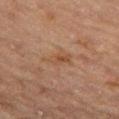This lesion was catalogued during total-body skin photography and was not selected for biopsy.
On the left thigh.
A female subject aged 53–57.
The lesion-visualizer software estimated an area of roughly 5.5 mm² and a shape eccentricity near 0.8. The analysis additionally found a lesion color around L≈43 a*≈17 b*≈29 in CIELAB, a lesion–skin lightness drop of about 5, and a normalized border contrast of about 5.5. It also reported a nevus-likeness score of about 0/100.
A region of skin cropped from a whole-body photographic capture, roughly 15 mm wide.
Captured under cross-polarized illumination.